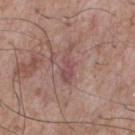Notes:
– body site · the chest
– image source · 15 mm crop, total-body photography
– patient · male, aged 73–77